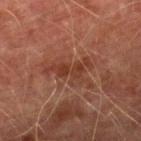No biopsy was performed on this lesion — it was imaged during a full skin examination and was not determined to be concerning. Automated image analysis of the tile measured a lesion area of about 8.5 mm², an outline eccentricity of about 0.85 (0 = round, 1 = elongated), and two-axis asymmetry of about 0.6. The analysis additionally found a classifier nevus-likeness of about 0/100 and lesion-presence confidence of about 85/100. From the leg. A male patient roughly 75 years of age. The lesion's longest dimension is about 5.5 mm. A close-up tile cropped from a whole-body skin photograph, about 15 mm across. Captured under cross-polarized illumination.